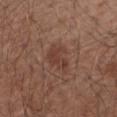Q: Is there a histopathology result?
A: imaged on a skin check; not biopsied